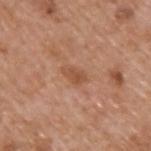Clinical summary:
A 15 mm crop from a total-body photograph taken for skin-cancer surveillance. On the upper back. A male subject, in their mid-60s.